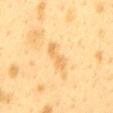Impression: No biopsy was performed on this lesion — it was imaged during a full skin examination and was not determined to be concerning. Background: Located on the mid back. A 15 mm close-up tile from a total-body photography series done for melanoma screening. A female patient, about 40 years old. Measured at roughly 3.5 mm in maximum diameter. Captured under cross-polarized illumination.